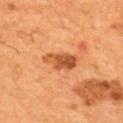Notes:
* biopsy status: total-body-photography surveillance lesion; no biopsy